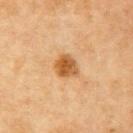Q: Was this lesion biopsied?
A: catalogued during a skin exam; not biopsied
Q: How was the tile lit?
A: cross-polarized illumination
Q: Where on the body is the lesion?
A: the left upper arm
Q: Who is the patient?
A: female, in their mid-50s
Q: What is the imaging modality?
A: ~15 mm crop, total-body skin-cancer survey